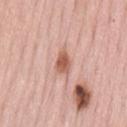Notes:
• diameter — ~3 mm (longest diameter)
• anatomic site — the lower back
• imaging modality — ~15 mm tile from a whole-body skin photo
• patient — female, roughly 70 years of age